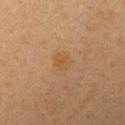{
  "biopsy_status": "not biopsied; imaged during a skin examination",
  "site": "left upper arm",
  "lesion_size": {
    "long_diameter_mm_approx": 2.5
  },
  "image": {
    "source": "total-body photography crop",
    "field_of_view_mm": 15
  },
  "patient": {
    "sex": "female",
    "age_approx": 40
  }
}